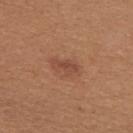Acquisition and patient details:
A female subject aged 38–42. On the upper back. This image is a 15 mm lesion crop taken from a total-body photograph.